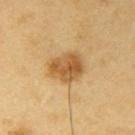Assessment:
The lesion was tiled from a total-body skin photograph and was not biopsied.
Context:
The lesion is on the right upper arm. A male patient, in their 60s. A region of skin cropped from a whole-body photographic capture, roughly 15 mm wide. The tile uses cross-polarized illumination.